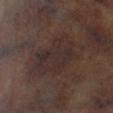biopsy_status: not biopsied; imaged during a skin examination
patient:
  sex: male
  age_approx: 65
image:
  source: total-body photography crop
  field_of_view_mm: 15
site: leg
lighting: cross-polarized